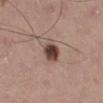Case summary:
- biopsy status — imaged on a skin check; not biopsied
- subject — male, aged 58 to 62
- image-analysis metrics — an area of roughly 7 mm² and two-axis asymmetry of about 0.15; a lesion color around L≈45 a*≈18 b*≈22 in CIELAB, about 15 CIELAB-L* units darker than the surrounding skin, and a normalized lesion–skin contrast near 11; a classifier nevus-likeness of about 95/100
- lesion size — ≈3 mm
- image source — ~15 mm tile from a whole-body skin photo
- illumination — white-light illumination
- location — the right lower leg